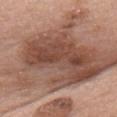The lesion was tiled from a total-body skin photograph and was not biopsied.
A female patient, aged approximately 60.
From the head or neck.
About 20 mm across.
The lesion-visualizer software estimated a footprint of about 120 mm², an outline eccentricity of about 0.9 (0 = round, 1 = elongated), and a symmetry-axis asymmetry near 0.35. The analysis additionally found an average lesion color of about L≈52 a*≈19 b*≈27 (CIELAB) and a lesion-to-skin contrast of about 9.5 (normalized; higher = more distinct). It also reported a nevus-likeness score of about 10/100.
The tile uses white-light illumination.
A lesion tile, about 15 mm wide, cut from a 3D total-body photograph.